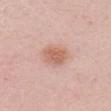The lesion was tiled from a total-body skin photograph and was not biopsied. The patient is a female roughly 20 years of age. Located on the head or neck. Captured under white-light illumination. Cropped from a whole-body photographic skin survey; the tile spans about 15 mm. Automated image analysis of the tile measured a lesion color around L≈62 a*≈22 b*≈30 in CIELAB and about 10 CIELAB-L* units darker than the surrounding skin. The analysis additionally found a border-irregularity rating of about 2/10 and peripheral color asymmetry of about 1. Measured at roughly 3.5 mm in maximum diameter.